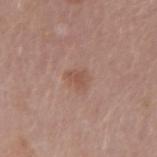Impression: The lesion was photographed on a routine skin check and not biopsied; there is no pathology result. Context: Located on the left forearm. This is a white-light tile. This image is a 15 mm lesion crop taken from a total-body photograph. The patient is a female in their mid- to late 40s. Measured at roughly 2.5 mm in maximum diameter.